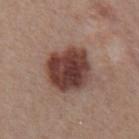biopsy_status: not biopsied; imaged during a skin examination
patient:
  sex: male
  age_approx: 55
automated_metrics:
  cielab_L: 39
  cielab_a: 21
  cielab_b: 23
  vs_skin_darker_L: 16.0
  border_irregularity_0_10: 1.5
  color_variation_0_10: 5.5
  peripheral_color_asymmetry: 2.0
image:
  source: total-body photography crop
  field_of_view_mm: 15
site: chest
lighting: white-light
lesion_size:
  long_diameter_mm_approx: 5.0